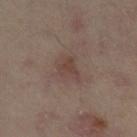Impression:
Part of a total-body skin-imaging series; this lesion was reviewed on a skin check and was not flagged for biopsy.
Clinical summary:
On the leg. Automated tile analysis of the lesion measured an eccentricity of roughly 0.45 and a shape-asymmetry score of about 0.4 (0 = symmetric). Imaged with cross-polarized lighting. A region of skin cropped from a whole-body photographic capture, roughly 15 mm wide. A female patient in their mid-30s. The lesion's longest dimension is about 2.5 mm.